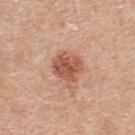A 15 mm crop from a total-body photograph taken for skin-cancer surveillance.
Imaged with white-light lighting.
A male patient aged approximately 60.
The lesion is located on the upper back.
Longest diameter approximately 3.5 mm.